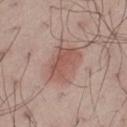Q: Was a biopsy performed?
A: imaged on a skin check; not biopsied
Q: What is the imaging modality?
A: ~15 mm crop, total-body skin-cancer survey
Q: What is the lesion's diameter?
A: ≈4.5 mm
Q: What is the anatomic site?
A: the leg
Q: What lighting was used for the tile?
A: white-light
Q: Patient demographics?
A: male, aged around 50